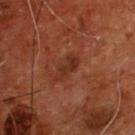Impression: Captured during whole-body skin photography for melanoma surveillance; the lesion was not biopsied. Clinical summary: The lesion is located on the chest. The total-body-photography lesion software estimated an area of roughly 5 mm², an eccentricity of roughly 0.9, and two-axis asymmetry of about 0.3. The subject is a male aged 53–57. Longest diameter approximately 4 mm. This is a cross-polarized tile. Cropped from a whole-body photographic skin survey; the tile spans about 15 mm.